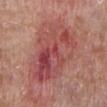This lesion was catalogued during total-body skin photography and was not selected for biopsy.